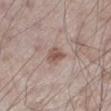follow-up = total-body-photography surveillance lesion; no biopsy | automated lesion analysis = a lesion color around L≈53 a*≈18 b*≈23 in CIELAB, about 10 CIELAB-L* units darker than the surrounding skin, and a normalized border contrast of about 7.5; a classifier nevus-likeness of about 65/100 | lesion diameter = ~2.5 mm (longest diameter) | body site = the left lower leg | patient = male, aged 58 to 62 | lighting = white-light | image = ~15 mm crop, total-body skin-cancer survey.